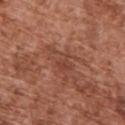Notes:
– notes: no biopsy performed (imaged during a skin exam)
– body site: the back
– patient: male, about 75 years old
– tile lighting: white-light illumination
– imaging modality: ~15 mm crop, total-body skin-cancer survey
– automated metrics: a lesion area of about 7.5 mm², a shape eccentricity near 0.85, and a symmetry-axis asymmetry near 0.45; a lesion–skin lightness drop of about 6 and a lesion-to-skin contrast of about 5 (normalized; higher = more distinct); a border-irregularity index near 6/10, internal color variation of about 4 on a 0–10 scale, and a peripheral color-asymmetry measure near 1.5; an automated nevus-likeness rating near 0 out of 100